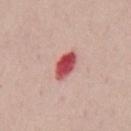Recorded during total-body skin imaging; not selected for excision or biopsy. Cropped from a total-body skin-imaging series; the visible field is about 15 mm. A male patient, about 50 years old. The recorded lesion diameter is about 3.5 mm. The lesion is on the chest. Imaged with white-light lighting.